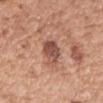Clinical summary: A 15 mm close-up tile from a total-body photography series done for melanoma screening. A female subject aged 58–62. From the right forearm.